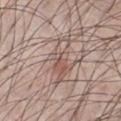Case summary:
• follow-up: imaged on a skin check; not biopsied
• automated lesion analysis: about 8 CIELAB-L* units darker than the surrounding skin and a lesion-to-skin contrast of about 5.5 (normalized; higher = more distinct)
• lesion size: about 5.5 mm
• anatomic site: the chest
• subject: male, aged around 40
• image source: 15 mm crop, total-body photography